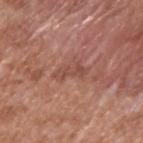The lesion was photographed on a routine skin check and not biopsied; there is no pathology result.
The lesion's longest dimension is about 4 mm.
A male patient, aged 63 to 67.
Located on the upper back.
A lesion tile, about 15 mm wide, cut from a 3D total-body photograph.